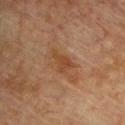Clinical impression:
Recorded during total-body skin imaging; not selected for excision or biopsy.
Acquisition and patient details:
A male patient, aged 73–77. This is a cross-polarized tile. The lesion is located on the chest. Cropped from a whole-body photographic skin survey; the tile spans about 15 mm.